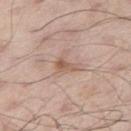Recorded during total-body skin imaging; not selected for excision or biopsy. This is a white-light tile. This image is a 15 mm lesion crop taken from a total-body photograph. A male patient about 55 years old. Located on the right thigh. Automated image analysis of the tile measured a border-irregularity rating of about 4.5/10 and a peripheral color-asymmetry measure near 0.5. It also reported an automated nevus-likeness rating near 0 out of 100 and a detector confidence of about 100 out of 100 that the crop contains a lesion. About 3 mm across.